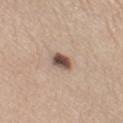The lesion was tiled from a total-body skin photograph and was not biopsied. An algorithmic analysis of the crop reported border irregularity of about 2 on a 0–10 scale, a within-lesion color-variation index near 4.5/10, and radial color variation of about 1.5. It also reported a nevus-likeness score of about 95/100. A region of skin cropped from a whole-body photographic capture, roughly 15 mm wide. From the leg. The lesion's longest dimension is about 2.5 mm. Imaged with white-light lighting. The patient is a female about 60 years old.